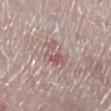Recorded during total-body skin imaging; not selected for excision or biopsy. The tile uses white-light illumination. Cropped from a total-body skin-imaging series; the visible field is about 15 mm. A female subject approximately 70 years of age. Approximately 3.5 mm at its widest. The lesion is located on the right lower leg.